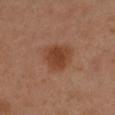Clinical impression: This lesion was catalogued during total-body skin photography and was not selected for biopsy.